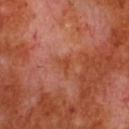No biopsy was performed on this lesion — it was imaged during a full skin examination and was not determined to be concerning. Cropped from a total-body skin-imaging series; the visible field is about 15 mm. A male subject, aged 53 to 57. From the chest.